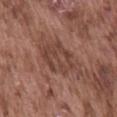follow-up — imaged on a skin check; not biopsied | diameter — ~5.5 mm (longest diameter) | site — the back | subject — male, aged approximately 75 | illumination — white-light illumination | imaging modality — 15 mm crop, total-body photography.